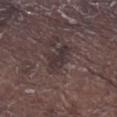The tile uses white-light illumination. Measured at roughly 4 mm in maximum diameter. On the right lower leg. A roughly 15 mm field-of-view crop from a total-body skin photograph. A male subject, approximately 70 years of age.